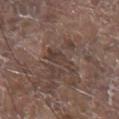Q: Was this lesion biopsied?
A: imaged on a skin check; not biopsied
Q: What lighting was used for the tile?
A: white-light illumination
Q: Where on the body is the lesion?
A: the chest
Q: What is the lesion's diameter?
A: ≈4 mm
Q: What is the imaging modality?
A: 15 mm crop, total-body photography
Q: What are the patient's age and sex?
A: male, aged around 80